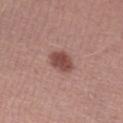Recorded during total-body skin imaging; not selected for excision or biopsy.
This image is a 15 mm lesion crop taken from a total-body photograph.
Automated image analysis of the tile measured an area of roughly 6.5 mm² and a symmetry-axis asymmetry near 0.25. It also reported an average lesion color of about L≈47 a*≈23 b*≈24 (CIELAB), roughly 13 lightness units darker than nearby skin, and a lesion-to-skin contrast of about 9 (normalized; higher = more distinct).
Measured at roughly 3.5 mm in maximum diameter.
The tile uses white-light illumination.
A male subject, approximately 30 years of age.